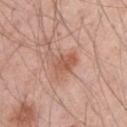Acquisition and patient details:
Located on the arm. This is a white-light tile. The lesion-visualizer software estimated an outline eccentricity of about 0.7 (0 = round, 1 = elongated) and a shape-asymmetry score of about 0.3 (0 = symmetric). It also reported a mean CIELAB color near L≈56 a*≈24 b*≈30, about 9 CIELAB-L* units darker than the surrounding skin, and a normalized lesion–skin contrast near 6.5. And it measured border irregularity of about 3 on a 0–10 scale, a color-variation rating of about 3/10, and peripheral color asymmetry of about 1. A male patient, in their mid-40s. A roughly 15 mm field-of-view crop from a total-body skin photograph. Approximately 3 mm at its widest.